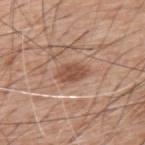subject: male, aged 58–62 | TBP lesion metrics: a footprint of about 6 mm² and a symmetry-axis asymmetry near 0.2; an average lesion color of about L≈51 a*≈22 b*≈29 (CIELAB) and roughly 11 lightness units darker than nearby skin; a classifier nevus-likeness of about 100/100 and a lesion-detection confidence of about 100/100 | size: ~3.5 mm (longest diameter) | illumination: white-light | location: the upper back | acquisition: total-body-photography crop, ~15 mm field of view.